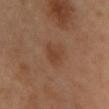follow-up — imaged on a skin check; not biopsied
patient — female, aged 38 to 42
body site — the chest
image-analysis metrics — an area of roughly 5.5 mm², a shape eccentricity near 0.65, and a symmetry-axis asymmetry near 0.2; a border-irregularity index near 2/10, internal color variation of about 1.5 on a 0–10 scale, and peripheral color asymmetry of about 0.5; a lesion-detection confidence of about 100/100
image — 15 mm crop, total-body photography